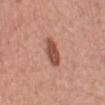The lesion was tiled from a total-body skin photograph and was not biopsied. Automated tile analysis of the lesion measured an eccentricity of roughly 0.8 and two-axis asymmetry of about 0.2. And it measured a color-variation rating of about 2.5/10 and a peripheral color-asymmetry measure near 1. The software also gave an automated nevus-likeness rating near 90 out of 100 and a lesion-detection confidence of about 100/100. Approximately 3.5 mm at its widest. Imaged with white-light lighting. The patient is a female roughly 65 years of age. A 15 mm crop from a total-body photograph taken for skin-cancer surveillance. The lesion is located on the left lower leg.